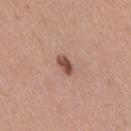Imaged during a routine full-body skin examination; the lesion was not biopsied and no histopathology is available. The lesion is on the right thigh. A region of skin cropped from a whole-body photographic capture, roughly 15 mm wide. A female subject approximately 40 years of age.